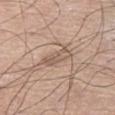Assessment:
The lesion was tiled from a total-body skin photograph and was not biopsied.
Context:
This is a white-light tile. A male subject, approximately 75 years of age. On the right thigh. About 4 mm across. A roughly 15 mm field-of-view crop from a total-body skin photograph.